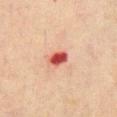Imaged with cross-polarized lighting.
A male patient, aged 68 to 72.
The lesion is on the mid back.
This image is a 15 mm lesion crop taken from a total-body photograph.
The lesion's longest dimension is about 2.5 mm.